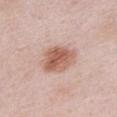Case summary:
– workup — imaged on a skin check; not biopsied
– imaging modality — total-body-photography crop, ~15 mm field of view
– body site — the chest
– lesion size — ~4.5 mm (longest diameter)
– patient — female, in their 30s
– lighting — white-light illumination
– image-analysis metrics — about 13 CIELAB-L* units darker than the surrounding skin and a normalized border contrast of about 8.5; a border-irregularity index near 2/10 and a within-lesion color-variation index near 5.5/10; a classifier nevus-likeness of about 100/100 and lesion-presence confidence of about 100/100